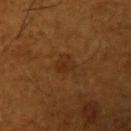Part of a total-body skin-imaging series; this lesion was reviewed on a skin check and was not flagged for biopsy. Located on the left upper arm. Automated tile analysis of the lesion measured peripheral color asymmetry of about 0.5. It also reported a lesion-detection confidence of about 100/100. The tile uses cross-polarized illumination. A male patient, in their mid- to late 60s. A close-up tile cropped from a whole-body skin photograph, about 15 mm across. The recorded lesion diameter is about 2.5 mm.